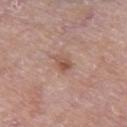notes: catalogued during a skin exam; not biopsied
subject: male, roughly 80 years of age
location: the right thigh
lighting: white-light
lesion size: ~2.5 mm (longest diameter)
image-analysis metrics: an outline eccentricity of about 0.8 (0 = round, 1 = elongated) and a shape-asymmetry score of about 0.25 (0 = symmetric); a border-irregularity rating of about 2.5/10; a classifier nevus-likeness of about 55/100
image source: ~15 mm tile from a whole-body skin photo